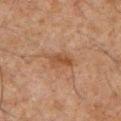{"biopsy_status": "not biopsied; imaged during a skin examination", "image": {"source": "total-body photography crop", "field_of_view_mm": 15}, "lighting": "cross-polarized", "site": "chest", "patient": {"sex": "male", "age_approx": 60}, "lesion_size": {"long_diameter_mm_approx": 3.0}}